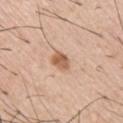No biopsy was performed on this lesion — it was imaged during a full skin examination and was not determined to be concerning. A 15 mm close-up tile from a total-body photography series done for melanoma screening. Longest diameter approximately 2.5 mm. A male subject, aged 53–57.